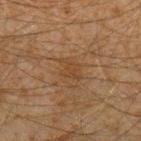Part of a total-body skin-imaging series; this lesion was reviewed on a skin check and was not flagged for biopsy. The recorded lesion diameter is about 3 mm. A male patient, aged around 35. On the arm. Imaged with cross-polarized lighting. A roughly 15 mm field-of-view crop from a total-body skin photograph.